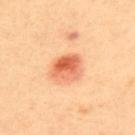Q: Was this lesion biopsied?
A: no biopsy performed (imaged during a skin exam)
Q: Where on the body is the lesion?
A: the upper back
Q: Patient demographics?
A: female, aged 38–42
Q: What kind of image is this?
A: ~15 mm crop, total-body skin-cancer survey
Q: Automated lesion metrics?
A: a nevus-likeness score of about 100/100